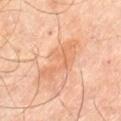<case>
<biopsy_status>not biopsied; imaged during a skin examination</biopsy_status>
<site>left thigh</site>
<lesion_size>
  <long_diameter_mm_approx>5.5</long_diameter_mm_approx>
</lesion_size>
<patient>
  <sex>male</sex>
  <age_approx>70</age_approx>
</patient>
<image>
  <source>total-body photography crop</source>
  <field_of_view_mm>15</field_of_view_mm>
</image>
<lighting>cross-polarized</lighting>
</case>